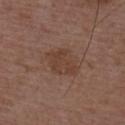The lesion was photographed on a routine skin check and not biopsied; there is no pathology result. On the upper back. Approximately 4 mm at its widest. The lesion-visualizer software estimated an area of roughly 9.5 mm², an eccentricity of roughly 0.65, and a symmetry-axis asymmetry near 0.15. And it measured a lesion color around L≈40 a*≈18 b*≈26 in CIELAB, a lesion–skin lightness drop of about 6, and a normalized lesion–skin contrast near 6. It also reported a border-irregularity rating of about 2/10. The analysis additionally found a classifier nevus-likeness of about 0/100. A 15 mm crop from a total-body photograph taken for skin-cancer surveillance. A male subject, approximately 50 years of age.